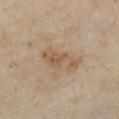<lesion>
  <biopsy_status>not biopsied; imaged during a skin examination</biopsy_status>
  <site>chest</site>
  <automated_metrics>
    <cielab_L>52</cielab_L>
    <cielab_a>15</cielab_a>
    <cielab_b>30</cielab_b>
    <vs_skin_darker_L>8.0</vs_skin_darker_L>
    <vs_skin_contrast_norm>6.5</vs_skin_contrast_norm>
  </automated_metrics>
  <image>
    <source>total-body photography crop</source>
    <field_of_view_mm>15</field_of_view_mm>
  </image>
  <patient>
    <sex>female</sex>
    <age_approx>35</age_approx>
  </patient>
  <lesion_size>
    <long_diameter_mm_approx>5.0</long_diameter_mm_approx>
  </lesion_size>
  <lighting>cross-polarized</lighting>
</lesion>